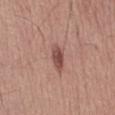The lesion was photographed on a routine skin check and not biopsied; there is no pathology result.
Approximately 3.5 mm at its widest.
A region of skin cropped from a whole-body photographic capture, roughly 15 mm wide.
A male patient about 55 years old.
Captured under white-light illumination.